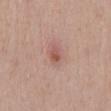<case>
  <biopsy_status>not biopsied; imaged during a skin examination</biopsy_status>
  <image>
    <source>total-body photography crop</source>
    <field_of_view_mm>15</field_of_view_mm>
  </image>
  <lighting>white-light</lighting>
  <site>mid back</site>
  <patient>
    <sex>male</sex>
    <age_approx>40</age_approx>
  </patient>
</case>